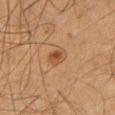The lesion was photographed on a routine skin check and not biopsied; there is no pathology result.
The patient is a male aged approximately 60.
The lesion is located on the lower back.
This image is a 15 mm lesion crop taken from a total-body photograph.